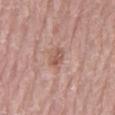<lesion>
<site>mid back</site>
<patient>
  <sex>male</sex>
  <age_approx>70</age_approx>
</patient>
<automated_metrics>
  <area_mm2_approx>3.0</area_mm2_approx>
  <shape_asymmetry>0.3</shape_asymmetry>
  <border_irregularity_0_10>3.0</border_irregularity_0_10>
  <peripheral_color_asymmetry>0.0</peripheral_color_asymmetry>
</automated_metrics>
<image>
  <source>total-body photography crop</source>
  <field_of_view_mm>15</field_of_view_mm>
</image>
</lesion>